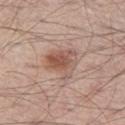<record>
  <biopsy_status>not biopsied; imaged during a skin examination</biopsy_status>
  <image>
    <source>total-body photography crop</source>
    <field_of_view_mm>15</field_of_view_mm>
  </image>
  <site>left thigh</site>
  <patient>
    <sex>male</sex>
    <age_approx>55</age_approx>
  </patient>
</record>